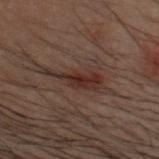This lesion was catalogued during total-body skin photography and was not selected for biopsy. The recorded lesion diameter is about 6.5 mm. The lesion is located on the head or neck. The subject is a male roughly 30 years of age. An algorithmic analysis of the crop reported an area of roughly 10 mm², an outline eccentricity of about 0.9 (0 = round, 1 = elongated), and a shape-asymmetry score of about 0.5 (0 = symmetric). This is a cross-polarized tile. A 15 mm close-up tile from a total-body photography series done for melanoma screening.